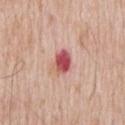Assessment:
Captured during whole-body skin photography for melanoma surveillance; the lesion was not biopsied.
Context:
The lesion is located on the back. A 15 mm close-up tile from a total-body photography series done for melanoma screening. Captured under white-light illumination. The patient is a male in their 60s. Approximately 3.5 mm at its widest.